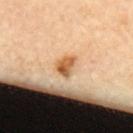{
  "biopsy_status": "not biopsied; imaged during a skin examination",
  "patient": {
    "sex": "female",
    "age_approx": 65
  },
  "image": {
    "source": "total-body photography crop",
    "field_of_view_mm": 15
  },
  "lighting": "cross-polarized",
  "site": "back",
  "lesion_size": {
    "long_diameter_mm_approx": 3.0
  }
}